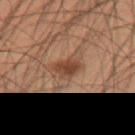Imaged during a routine full-body skin examination; the lesion was not biopsied and no histopathology is available. This is a cross-polarized tile. Cropped from a whole-body photographic skin survey; the tile spans about 15 mm. A male subject aged 53 to 57. Longest diameter approximately 3 mm. Automated image analysis of the tile measured an average lesion color of about L≈34 a*≈18 b*≈25 (CIELAB). The lesion is located on the right thigh.